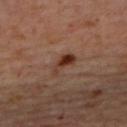  biopsy_status: not biopsied; imaged during a skin examination
  lesion_size:
    long_diameter_mm_approx: 3.5
  lighting: cross-polarized
  automated_metrics:
    color_variation_0_10: 4.5
  site: upper back
  patient:
    sex: female
    age_approx: 45
  image:
    source: total-body photography crop
    field_of_view_mm: 15